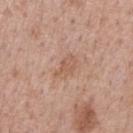| feature | finding |
|---|---|
| image source | 15 mm crop, total-body photography |
| site | the right forearm |
| illumination | white-light illumination |
| lesion size | ~3 mm (longest diameter) |
| patient | female, in their 40s |
| image-analysis metrics | a shape-asymmetry score of about 0.3 (0 = symmetric); an average lesion color of about L≈57 a*≈20 b*≈30 (CIELAB) and a normalized border contrast of about 5; a border-irregularity index near 3/10 and a within-lesion color-variation index near 1.5/10 |